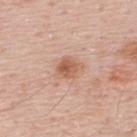illumination: white-light | acquisition: ~15 mm crop, total-body skin-cancer survey | anatomic site: the upper back | subject: male, aged around 50 | automated lesion analysis: an outline eccentricity of about 0.35 (0 = round, 1 = elongated); roughly 10 lightness units darker than nearby skin; a border-irregularity index near 2.5/10, internal color variation of about 4.5 on a 0–10 scale, and a peripheral color-asymmetry measure near 1.5 | diameter: ~2.5 mm (longest diameter).